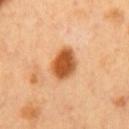patient:
  sex: male
  age_approx: 50
lighting: cross-polarized
lesion_size:
  long_diameter_mm_approx: 4.0
site: front of the torso
image:
  source: total-body photography crop
  field_of_view_mm: 15